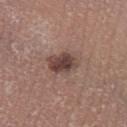The lesion was photographed on a routine skin check and not biopsied; there is no pathology result. A female subject, roughly 30 years of age. This is a white-light tile. The lesion is located on the left lower leg. A 15 mm close-up tile from a total-body photography series done for melanoma screening.